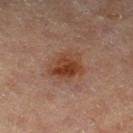Impression: This lesion was catalogued during total-body skin photography and was not selected for biopsy. Clinical summary: Approximately 4 mm at its widest. This is a cross-polarized tile. The patient is a male in their mid- to late 80s or older. A region of skin cropped from a whole-body photographic capture, roughly 15 mm wide. From the left thigh.